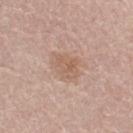Image and clinical context: The tile uses white-light illumination. Automated image analysis of the tile measured a lesion color around L≈60 a*≈18 b*≈28 in CIELAB, roughly 7 lightness units darker than nearby skin, and a normalized border contrast of about 5.5. It also reported a border-irregularity rating of about 2.5/10, a color-variation rating of about 3/10, and a peripheral color-asymmetry measure near 1. A 15 mm close-up tile from a total-body photography series done for melanoma screening. A female subject, in their 70s. From the left thigh. Measured at roughly 4 mm in maximum diameter.